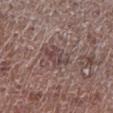Q: Was a biopsy performed?
A: catalogued during a skin exam; not biopsied
Q: How large is the lesion?
A: about 4 mm
Q: What is the imaging modality?
A: ~15 mm crop, total-body skin-cancer survey
Q: Who is the patient?
A: male, roughly 70 years of age
Q: Where on the body is the lesion?
A: the left lower leg
Q: Automated lesion metrics?
A: roughly 8 lightness units darker than nearby skin and a normalized lesion–skin contrast near 6.5; an automated nevus-likeness rating near 0 out of 100 and a detector confidence of about 75 out of 100 that the crop contains a lesion
Q: How was the tile lit?
A: white-light